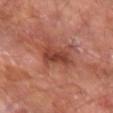imaging modality: 15 mm crop, total-body photography
patient: male, in their mid-60s
automated lesion analysis: a shape-asymmetry score of about 0.25 (0 = symmetric)
illumination: cross-polarized illumination
body site: the left forearm
diameter: ~3.5 mm (longest diameter)